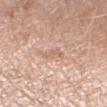Captured during whole-body skin photography for melanoma surveillance; the lesion was not biopsied. A roughly 15 mm field-of-view crop from a total-body skin photograph. A female patient aged 58 to 62. Located on the left forearm. An algorithmic analysis of the crop reported an average lesion color of about L≈64 a*≈19 b*≈28 (CIELAB), about 7 CIELAB-L* units darker than the surrounding skin, and a normalized lesion–skin contrast near 4.5. The software also gave a border-irregularity index near 4.5/10. It also reported a classifier nevus-likeness of about 0/100 and a detector confidence of about 95 out of 100 that the crop contains a lesion.